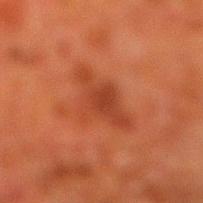The lesion was photographed on a routine skin check and not biopsied; there is no pathology result.
The lesion is on the right lower leg.
Imaged with cross-polarized lighting.
Automated tile analysis of the lesion measured a lesion area of about 12 mm² and a symmetry-axis asymmetry near 0.4. And it measured a lesion color around L≈34 a*≈27 b*≈31 in CIELAB, roughly 7 lightness units darker than nearby skin, and a lesion-to-skin contrast of about 6 (normalized; higher = more distinct).
A 15 mm close-up tile from a total-body photography series done for melanoma screening.
The patient is a male aged around 80.